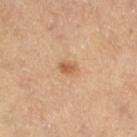Imaged during a routine full-body skin examination; the lesion was not biopsied and no histopathology is available.
The subject is a female aged 63–67.
The lesion is located on the right thigh.
Longest diameter approximately 2.5 mm.
Captured under cross-polarized illumination.
A close-up tile cropped from a whole-body skin photograph, about 15 mm across.